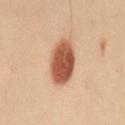| field | value |
|---|---|
| workup | total-body-photography surveillance lesion; no biopsy |
| diameter | ≈5 mm |
| subject | female, aged 28 to 32 |
| site | the lower back |
| automated metrics | a shape eccentricity near 0.7 and a shape-asymmetry score of about 0.05 (0 = symmetric); border irregularity of about 1 on a 0–10 scale, a color-variation rating of about 4/10, and a peripheral color-asymmetry measure near 1.5; a classifier nevus-likeness of about 100/100 and lesion-presence confidence of about 100/100 |
| acquisition | ~15 mm tile from a whole-body skin photo |
| illumination | cross-polarized illumination |